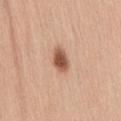| key | value |
|---|---|
| follow-up | imaged on a skin check; not biopsied |
| imaging modality | total-body-photography crop, ~15 mm field of view |
| site | the back |
| automated lesion analysis | a footprint of about 5.5 mm², an eccentricity of roughly 0.8, and a shape-asymmetry score of about 0.2 (0 = symmetric) |
| lesion diameter | about 3.5 mm |
| patient | female, aged approximately 45 |